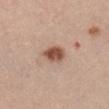Notes:
* follow-up · imaged on a skin check; not biopsied